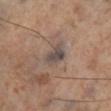Imaged during a routine full-body skin examination; the lesion was not biopsied and no histopathology is available. This image is a 15 mm lesion crop taken from a total-body photograph. On the leg.